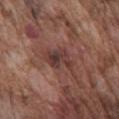{"biopsy_status": "not biopsied; imaged during a skin examination", "site": "front of the torso", "lesion_size": {"long_diameter_mm_approx": 4.5}, "lighting": "white-light", "image": {"source": "total-body photography crop", "field_of_view_mm": 15}, "patient": {"sex": "male", "age_approx": 75}}